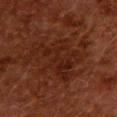Imaged during a routine full-body skin examination; the lesion was not biopsied and no histopathology is available. A female subject roughly 50 years of age. The tile uses cross-polarized illumination. From the upper back. About 6.5 mm across. Cropped from a total-body skin-imaging series; the visible field is about 15 mm.